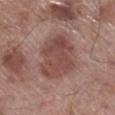Recorded during total-body skin imaging; not selected for excision or biopsy. A male patient roughly 70 years of age. On the leg. A 15 mm close-up extracted from a 3D total-body photography capture.